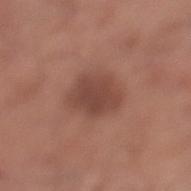No biopsy was performed on this lesion — it was imaged during a full skin examination and was not determined to be concerning. The recorded lesion diameter is about 4 mm. A male patient approximately 65 years of age. Located on the leg. A 15 mm close-up extracted from a 3D total-body photography capture.